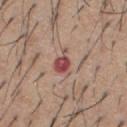Q: Is there a histopathology result?
A: catalogued during a skin exam; not biopsied
Q: Automated lesion metrics?
A: an average lesion color of about L≈49 a*≈25 b*≈23 (CIELAB) and a lesion-to-skin contrast of about 9 (normalized; higher = more distinct); border irregularity of about 4.5 on a 0–10 scale and peripheral color asymmetry of about 1.5
Q: Patient demographics?
A: male, in their 60s
Q: How was this image acquired?
A: ~15 mm tile from a whole-body skin photo
Q: How large is the lesion?
A: about 3.5 mm
Q: What lighting was used for the tile?
A: white-light illumination
Q: Lesion location?
A: the chest